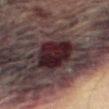Case summary:
– subject · female, approximately 80 years of age
– body site · the left leg
– image · ~15 mm crop, total-body skin-cancer survey
– lesion size · about 6 mm
– lighting · cross-polarized